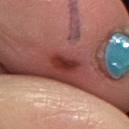{"biopsy_status": "not biopsied; imaged during a skin examination", "patient": {"sex": "female", "age_approx": 55}, "automated_metrics": {"border_irregularity_0_10": 3.0, "color_variation_0_10": 3.5, "peripheral_color_asymmetry": 1.0}, "image": {"source": "total-body photography crop", "field_of_view_mm": 15}, "site": "leg", "lesion_size": {"long_diameter_mm_approx": 3.0}}